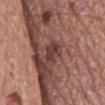{
  "biopsy_status": "not biopsied; imaged during a skin examination"
}